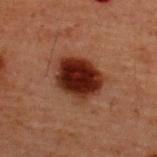Q: Is there a histopathology result?
A: imaged on a skin check; not biopsied
Q: What kind of image is this?
A: ~15 mm crop, total-body skin-cancer survey
Q: What is the anatomic site?
A: the upper back
Q: Lesion size?
A: ≈5 mm
Q: What are the patient's age and sex?
A: male, aged 58 to 62
Q: Automated lesion metrics?
A: a lesion area of about 18 mm², a shape eccentricity near 0.55, and a shape-asymmetry score of about 0.2 (0 = symmetric); a nevus-likeness score of about 100/100 and lesion-presence confidence of about 100/100